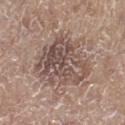This lesion was catalogued during total-body skin photography and was not selected for biopsy. The subject is a male aged around 70. An algorithmic analysis of the crop reported border irregularity of about 4.5 on a 0–10 scale and a color-variation rating of about 7/10. Approximately 7 mm at its widest. Captured under white-light illumination. The lesion is located on the right lower leg. A lesion tile, about 15 mm wide, cut from a 3D total-body photograph.